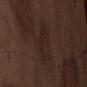Impression:
The lesion was photographed on a routine skin check and not biopsied; there is no pathology result.